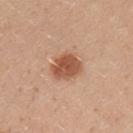biopsy_status: not biopsied; imaged during a skin examination
site: left upper arm
lighting: white-light
lesion_size:
  long_diameter_mm_approx: 3.5
image:
  source: total-body photography crop
  field_of_view_mm: 15
patient:
  sex: male
  age_approx: 35
automated_metrics:
  border_irregularity_0_10: 1.5
  color_variation_0_10: 3.0
  nevus_likeness_0_100: 100